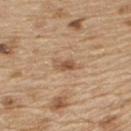biopsy status = imaged on a skin check; not biopsied
body site = the upper back
lesion size = about 3 mm
image-analysis metrics = a lesion color around L≈54 a*≈18 b*≈33 in CIELAB, roughly 10 lightness units darker than nearby skin, and a normalized border contrast of about 7; lesion-presence confidence of about 100/100
tile lighting = white-light
subject = male, approximately 70 years of age
image = ~15 mm crop, total-body skin-cancer survey